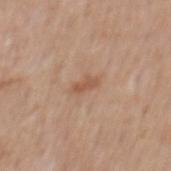| key | value |
|---|---|
| site | the mid back |
| tile lighting | white-light illumination |
| lesion diameter | ~2.5 mm (longest diameter) |
| patient | male, in their mid-60s |
| imaging modality | 15 mm crop, total-body photography |